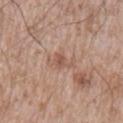Clinical impression:
The lesion was photographed on a routine skin check and not biopsied; there is no pathology result.
Background:
An algorithmic analysis of the crop reported a lesion color around L≈54 a*≈20 b*≈27 in CIELAB, about 8 CIELAB-L* units darker than the surrounding skin, and a normalized border contrast of about 5.5. It also reported a classifier nevus-likeness of about 0/100. Captured under white-light illumination. Located on the left upper arm. The lesion's longest dimension is about 2.5 mm. A region of skin cropped from a whole-body photographic capture, roughly 15 mm wide. The subject is a male in their mid-60s.